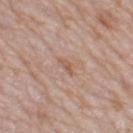| field | value |
|---|---|
| patient | female, roughly 60 years of age |
| location | the left thigh |
| diameter | ≈2.5 mm |
| image source | ~15 mm crop, total-body skin-cancer survey |
| tile lighting | white-light illumination |